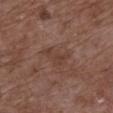{"biopsy_status": "not biopsied; imaged during a skin examination", "site": "chest", "image": {"source": "total-body photography crop", "field_of_view_mm": 15}, "patient": {"sex": "female", "age_approx": 80}, "lighting": "white-light"}